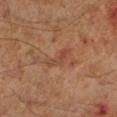subject: male, aged 58–62 | location: the left lower leg | diameter: ≈4 mm | tile lighting: cross-polarized | imaging modality: total-body-photography crop, ~15 mm field of view.